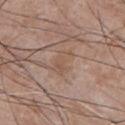| key | value |
|---|---|
| workup | imaged on a skin check; not biopsied |
| acquisition | total-body-photography crop, ~15 mm field of view |
| patient | male, roughly 50 years of age |
| anatomic site | the chest |